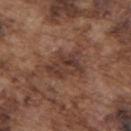The lesion was tiled from a total-body skin photograph and was not biopsied.
Automated image analysis of the tile measured a border-irregularity index near 5/10 and a peripheral color-asymmetry measure near 2. The software also gave a nevus-likeness score of about 10/100 and a lesion-detection confidence of about 95/100.
The patient is a male aged approximately 75.
On the upper back.
A lesion tile, about 15 mm wide, cut from a 3D total-body photograph.
The lesion's longest dimension is about 5 mm.
The tile uses white-light illumination.